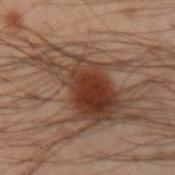acquisition: ~15 mm crop, total-body skin-cancer survey
location: the left arm
image-analysis metrics: a shape-asymmetry score of about 0.4 (0 = symmetric); a mean CIELAB color near L≈31 a*≈16 b*≈22 and a lesion-to-skin contrast of about 10 (normalized; higher = more distinct); a border-irregularity rating of about 7.5/10, a within-lesion color-variation index near 8.5/10, and peripheral color asymmetry of about 2.5; a nevus-likeness score of about 95/100 and a detector confidence of about 100 out of 100 that the crop contains a lesion
patient: male, aged 48–52
size: about 9.5 mm
tile lighting: cross-polarized